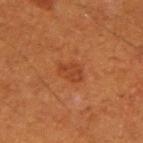Part of a total-body skin-imaging series; this lesion was reviewed on a skin check and was not flagged for biopsy. Cropped from a total-body skin-imaging series; the visible field is about 15 mm. Imaged with cross-polarized lighting. A male subject roughly 60 years of age. From the right upper arm.